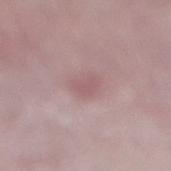  biopsy_status: not biopsied; imaged during a skin examination
  patient:
    sex: female
    age_approx: 65
  image:
    source: total-body photography crop
    field_of_view_mm: 15
  site: right lower leg